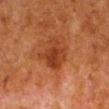biopsy_status: not biopsied; imaged during a skin examination
lighting: cross-polarized
site: right lower leg
lesion_size:
  long_diameter_mm_approx: 3.5
automated_metrics:
  area_mm2_approx: 10.0
  eccentricity: 0.35
  shape_asymmetry: 0.3
  cielab_L: 31
  cielab_a: 24
  cielab_b: 31
  vs_skin_darker_L: 8.0
  vs_skin_contrast_norm: 7.5
image:
  source: total-body photography crop
  field_of_view_mm: 15
patient:
  sex: male
  age_approx: 80